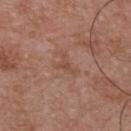Assessment:
No biopsy was performed on this lesion — it was imaged during a full skin examination and was not determined to be concerning.
Context:
Cropped from a whole-body photographic skin survey; the tile spans about 15 mm. A male subject, aged 53 to 57. Located on the chest. Automated image analysis of the tile measured border irregularity of about 7.5 on a 0–10 scale, internal color variation of about 0 on a 0–10 scale, and a peripheral color-asymmetry measure near 0. It also reported a nevus-likeness score of about 0/100 and lesion-presence confidence of about 100/100. Approximately 2.5 mm at its widest.